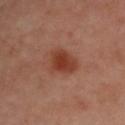Clinical impression:
The lesion was tiled from a total-body skin photograph and was not biopsied.
Background:
A 15 mm close-up tile from a total-body photography series done for melanoma screening. A female subject roughly 50 years of age. The lesion-visualizer software estimated a mean CIELAB color near L≈41 a*≈27 b*≈31, about 10 CIELAB-L* units darker than the surrounding skin, and a normalized lesion–skin contrast near 9. And it measured a border-irregularity rating of about 3/10 and internal color variation of about 3 on a 0–10 scale. Approximately 4.5 mm at its widest. Captured under cross-polarized illumination. From the right upper arm.